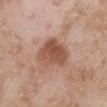| field | value |
|---|---|
| biopsy status | catalogued during a skin exam; not biopsied |
| patient | male, about 50 years old |
| site | the right upper arm |
| lighting | white-light illumination |
| size | ≈5 mm |
| image source | ~15 mm tile from a whole-body skin photo |